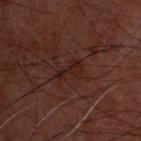Imaged during a routine full-body skin examination; the lesion was not biopsied and no histopathology is available.